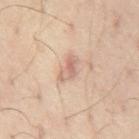Assessment:
Part of a total-body skin-imaging series; this lesion was reviewed on a skin check and was not flagged for biopsy.
Acquisition and patient details:
A region of skin cropped from a whole-body photographic capture, roughly 15 mm wide. The lesion is on the abdomen. The subject is a male about 60 years old. Captured under cross-polarized illumination.